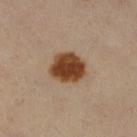Part of a total-body skin-imaging series; this lesion was reviewed on a skin check and was not flagged for biopsy.
A female subject, aged 38 to 42.
The lesion is located on the right lower leg.
A region of skin cropped from a whole-body photographic capture, roughly 15 mm wide.